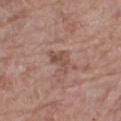Captured during whole-body skin photography for melanoma surveillance; the lesion was not biopsied.
Automated image analysis of the tile measured a footprint of about 5 mm², an outline eccentricity of about 0.75 (0 = round, 1 = elongated), and a shape-asymmetry score of about 0.45 (0 = symmetric). The analysis additionally found a lesion color around L≈50 a*≈20 b*≈25 in CIELAB and about 8 CIELAB-L* units darker than the surrounding skin. The software also gave border irregularity of about 4.5 on a 0–10 scale, internal color variation of about 2.5 on a 0–10 scale, and radial color variation of about 1.
Longest diameter approximately 3.5 mm.
On the right thigh.
This image is a 15 mm lesion crop taken from a total-body photograph.
The patient is a female aged 68 to 72.
Captured under white-light illumination.